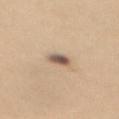{
  "biopsy_status": "not biopsied; imaged during a skin examination",
  "image": {
    "source": "total-body photography crop",
    "field_of_view_mm": 15
  },
  "lesion_size": {
    "long_diameter_mm_approx": 3.0
  },
  "lighting": "white-light",
  "site": "mid back",
  "patient": {
    "sex": "female",
    "age_approx": 30
  }
}